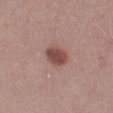follow-up: no biopsy performed (imaged during a skin exam)
lighting: white-light illumination
patient: female, about 40 years old
lesion size: ≈3 mm
TBP lesion metrics: a lesion area of about 6.5 mm², an eccentricity of roughly 0.65, and a shape-asymmetry score of about 0.2 (0 = symmetric); an average lesion color of about L≈47 a*≈22 b*≈23 (CIELAB), roughly 12 lightness units darker than nearby skin, and a normalized lesion–skin contrast near 9; border irregularity of about 1.5 on a 0–10 scale, a within-lesion color-variation index near 2/10, and a peripheral color-asymmetry measure near 0.5
image source: ~15 mm crop, total-body skin-cancer survey
location: the right lower leg